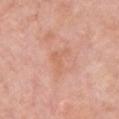Impression:
The lesion was tiled from a total-body skin photograph and was not biopsied.
Context:
This image is a 15 mm lesion crop taken from a total-body photograph. Located on the front of the torso. A female patient aged approximately 60.